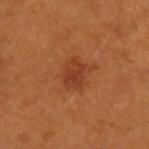Assessment:
This lesion was catalogued during total-body skin photography and was not selected for biopsy.
Context:
Cropped from a total-body skin-imaging series; the visible field is about 15 mm. A male subject approximately 55 years of age. The lesion is on the left upper arm.